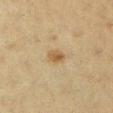A female patient aged 38–42. The lesion is located on the left lower leg. A roughly 15 mm field-of-view crop from a total-body skin photograph.